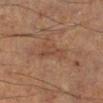follow-up=catalogued during a skin exam; not biopsied | imaging modality=~15 mm tile from a whole-body skin photo | anatomic site=the leg | illumination=cross-polarized illumination | lesion diameter=~4 mm (longest diameter) | subject=male, aged around 55 | automated lesion analysis=an area of roughly 5 mm², an eccentricity of roughly 0.9, and two-axis asymmetry of about 0.55; border irregularity of about 6 on a 0–10 scale, a within-lesion color-variation index near 2/10, and peripheral color asymmetry of about 1.